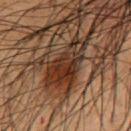Q: Is there a histopathology result?
A: imaged on a skin check; not biopsied
Q: What lighting was used for the tile?
A: cross-polarized
Q: Patient demographics?
A: male, aged 43 to 47
Q: What kind of image is this?
A: ~15 mm tile from a whole-body skin photo
Q: How large is the lesion?
A: ~6.5 mm (longest diameter)
Q: Lesion location?
A: the chest